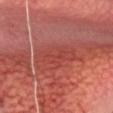Longest diameter approximately 5.5 mm. The tile uses cross-polarized illumination. A roughly 15 mm field-of-view crop from a total-body skin photograph. From the head or neck. The lesion-visualizer software estimated a lesion color around L≈45 a*≈34 b*≈28 in CIELAB, a lesion–skin lightness drop of about 6, and a normalized lesion–skin contrast near 4.5. A male subject, roughly 50 years of age.